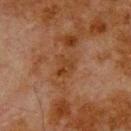Recorded during total-body skin imaging; not selected for excision or biopsy. On the upper back. The total-body-photography lesion software estimated an average lesion color of about L≈31 a*≈18 b*≈29 (CIELAB), a lesion–skin lightness drop of about 5, and a lesion-to-skin contrast of about 6.5 (normalized; higher = more distinct). Longest diameter approximately 2.5 mm. A 15 mm close-up tile from a total-body photography series done for melanoma screening. The subject is a male approximately 80 years of age.